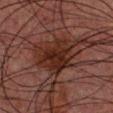Clinical summary:
A 15 mm close-up tile from a total-body photography series done for melanoma screening. A male subject, aged around 30. This is a cross-polarized tile. The lesion's longest dimension is about 5.5 mm. The lesion is on the chest.